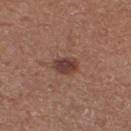workup=catalogued during a skin exam; not biopsied.